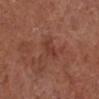<record>
<biopsy_status>not biopsied; imaged during a skin examination</biopsy_status>
<lesion_size>
  <long_diameter_mm_approx>2.5</long_diameter_mm_approx>
</lesion_size>
<automated_metrics>
  <area_mm2_approx>3.5</area_mm2_approx>
  <eccentricity>0.75</eccentricity>
  <shape_asymmetry>0.4</shape_asymmetry>
  <vs_skin_contrast_norm>5.5</vs_skin_contrast_norm>
</automated_metrics>
<site>left lower leg</site>
<patient>
  <sex>male</sex>
  <age_approx>75</age_approx>
</patient>
<image>
  <source>total-body photography crop</source>
  <field_of_view_mm>15</field_of_view_mm>
</image>
</record>